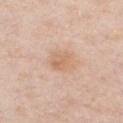Q: Was a biopsy performed?
A: catalogued during a skin exam; not biopsied
Q: Who is the patient?
A: male, in their 50s
Q: How was this image acquired?
A: ~15 mm tile from a whole-body skin photo
Q: Lesion size?
A: ~3 mm (longest diameter)
Q: Where on the body is the lesion?
A: the chest